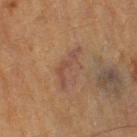Part of a total-body skin-imaging series; this lesion was reviewed on a skin check and was not flagged for biopsy. The lesion-visualizer software estimated a lesion area of about 8.5 mm², an eccentricity of roughly 0.9, and two-axis asymmetry of about 0.35. It also reported a within-lesion color-variation index near 3.5/10 and a peripheral color-asymmetry measure near 1.5. The analysis additionally found a classifier nevus-likeness of about 5/100 and a lesion-detection confidence of about 50/100. The patient is a male aged around 85 or older. Longest diameter approximately 5.5 mm. On the left thigh. A roughly 15 mm field-of-view crop from a total-body skin photograph.